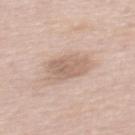Part of a total-body skin-imaging series; this lesion was reviewed on a skin check and was not flagged for biopsy. Located on the mid back. The tile uses white-light illumination. Cropped from a whole-body photographic skin survey; the tile spans about 15 mm. The lesion-visualizer software estimated a border-irregularity index near 2.5/10, a color-variation rating of about 3/10, and a peripheral color-asymmetry measure near 1. It also reported a detector confidence of about 100 out of 100 that the crop contains a lesion. A male patient, aged around 55. About 3.5 mm across.